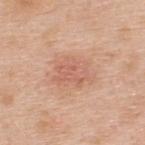The lesion was photographed on a routine skin check and not biopsied; there is no pathology result. The lesion is on the upper back. A lesion tile, about 15 mm wide, cut from a 3D total-body photograph. This is a white-light tile. The lesion's longest dimension is about 4.5 mm. A male subject, in their 50s.